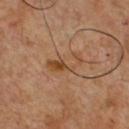biopsy status = imaged on a skin check; not biopsied
diameter = about 4.5 mm
location = the chest
image source = ~15 mm tile from a whole-body skin photo
image-analysis metrics = a footprint of about 6.5 mm² and two-axis asymmetry of about 0.45; a lesion color around L≈47 a*≈20 b*≈34 in CIELAB and roughly 8 lightness units darker than nearby skin
illumination = cross-polarized illumination
subject = male, aged approximately 50